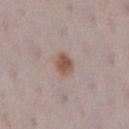biopsy status: no biopsy performed (imaged during a skin exam) | image source: ~15 mm crop, total-body skin-cancer survey | lesion diameter: ~3 mm (longest diameter) | location: the right lower leg | TBP lesion metrics: a lesion area of about 5.5 mm², a shape eccentricity near 0.6, and a symmetry-axis asymmetry near 0.2; a mean CIELAB color near L≈54 a*≈17 b*≈25 and roughly 10 lightness units darker than nearby skin; border irregularity of about 2 on a 0–10 scale and peripheral color asymmetry of about 1 | subject: female, aged around 30 | lighting: white-light.